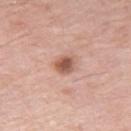No biopsy was performed on this lesion — it was imaged during a full skin examination and was not determined to be concerning. This image is a 15 mm lesion crop taken from a total-body photograph. This is a white-light tile. Measured at roughly 2.5 mm in maximum diameter. On the right thigh. The subject is a male in their 80s.